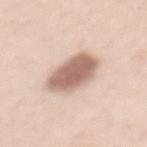notes=catalogued during a skin exam; not biopsied | TBP lesion metrics=an average lesion color of about L≈63 a*≈19 b*≈26 (CIELAB) and a normalized border contrast of about 10; a nevus-likeness score of about 95/100 and lesion-presence confidence of about 100/100 | subject=female, roughly 40 years of age | image source=total-body-photography crop, ~15 mm field of view | location=the back | tile lighting=white-light illumination.